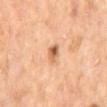| feature | finding |
|---|---|
| automated metrics | an area of roughly 3.5 mm², a shape eccentricity near 0.8, and a symmetry-axis asymmetry near 0.3; a mean CIELAB color near L≈61 a*≈23 b*≈37 and a normalized border contrast of about 8.5; a border-irregularity rating of about 2.5/10, a within-lesion color-variation index near 8.5/10, and radial color variation of about 3; a classifier nevus-likeness of about 90/100 |
| lesion diameter | ≈2.5 mm |
| patient | male, aged 68 to 72 |
| body site | the mid back |
| acquisition | 15 mm crop, total-body photography |